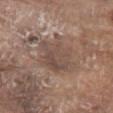Notes:
* workup: catalogued during a skin exam; not biopsied
* patient: male, about 80 years old
* body site: the abdomen
* tile lighting: white-light illumination
* imaging modality: ~15 mm crop, total-body skin-cancer survey
* size: about 5.5 mm